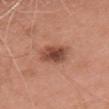follow-up — catalogued during a skin exam; not biopsied | diameter — ≈4 mm | image source — ~15 mm tile from a whole-body skin photo | patient — female, in their mid-60s | automated lesion analysis — a lesion area of about 8.5 mm², an eccentricity of roughly 0.85, and two-axis asymmetry of about 0.2; a mean CIELAB color near L≈48 a*≈25 b*≈29, a lesion–skin lightness drop of about 13, and a normalized lesion–skin contrast near 9; border irregularity of about 2 on a 0–10 scale, a color-variation rating of about 5/10, and a peripheral color-asymmetry measure near 1.5; a nevus-likeness score of about 85/100 and lesion-presence confidence of about 100/100 | anatomic site — the head or neck | illumination — white-light.